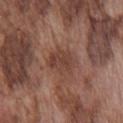Part of a total-body skin-imaging series; this lesion was reviewed on a skin check and was not flagged for biopsy. The lesion's longest dimension is about 3.5 mm. Captured under white-light illumination. From the chest. A 15 mm close-up extracted from a 3D total-body photography capture. A male subject aged 73 to 77.